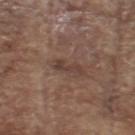<tbp_lesion>
<biopsy_status>not biopsied; imaged during a skin examination</biopsy_status>
<lesion_size>
  <long_diameter_mm_approx>3.0</long_diameter_mm_approx>
</lesion_size>
<automated_metrics>
  <area_mm2_approx>3.5</area_mm2_approx>
  <eccentricity>0.9</eccentricity>
  <shape_asymmetry>0.35</shape_asymmetry>
  <cielab_L>39</cielab_L>
  <cielab_a>17</cielab_a>
  <cielab_b>23</cielab_b>
  <vs_skin_darker_L>7.0</vs_skin_darker_L>
  <vs_skin_contrast_norm>6.5</vs_skin_contrast_norm>
  <border_irregularity_0_10>5.0</border_irregularity_0_10>
  <color_variation_0_10>0.0</color_variation_0_10>
  <peripheral_color_asymmetry>0.0</peripheral_color_asymmetry>
  <nevus_likeness_0_100>0</nevus_likeness_0_100>
  <lesion_detection_confidence_0_100>100</lesion_detection_confidence_0_100>
</automated_metrics>
<site>head or neck</site>
<patient>
  <sex>male</sex>
  <age_approx>80</age_approx>
</patient>
<image>
  <source>total-body photography crop</source>
  <field_of_view_mm>15</field_of_view_mm>
</image>
<lighting>white-light</lighting>
</tbp_lesion>